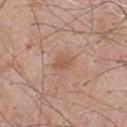Notes:
- workup: catalogued during a skin exam; not biopsied
- subject: male, approximately 65 years of age
- illumination: white-light
- automated metrics: a lesion area of about 4 mm², an outline eccentricity of about 0.75 (0 = round, 1 = elongated), and a shape-asymmetry score of about 0.25 (0 = symmetric); a lesion color around L≈55 a*≈20 b*≈31 in CIELAB and about 7 CIELAB-L* units darker than the surrounding skin; a lesion-detection confidence of about 100/100
- anatomic site: the chest
- image: ~15 mm tile from a whole-body skin photo